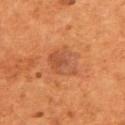workup = no biopsy performed (imaged during a skin exam)
body site = the upper back
image = ~15 mm crop, total-body skin-cancer survey
automated lesion analysis = a within-lesion color-variation index near 4.5/10 and peripheral color asymmetry of about 1.5; an automated nevus-likeness rating near 20 out of 100 and a lesion-detection confidence of about 100/100
subject = male, aged 53–57